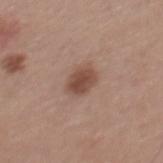Q: What is the lesion's diameter?
A: ≈3 mm
Q: What are the patient's age and sex?
A: male, in their mid-50s
Q: What did automated image analysis measure?
A: a classifier nevus-likeness of about 95/100 and a detector confidence of about 100 out of 100 that the crop contains a lesion
Q: What kind of image is this?
A: ~15 mm tile from a whole-body skin photo
Q: What is the anatomic site?
A: the mid back
Q: What lighting was used for the tile?
A: white-light